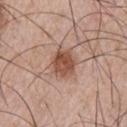Findings:
* notes — catalogued during a skin exam; not biopsied
* subject — male, roughly 55 years of age
* lighting — white-light illumination
* image source — 15 mm crop, total-body photography
* location — the left upper arm